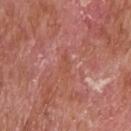anatomic site — the head or neck | illumination — white-light | patient — male, aged 63–67 | lesion size — about 2.5 mm | imaging modality — ~15 mm crop, total-body skin-cancer survey.